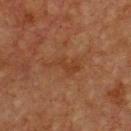Case summary:
* workup: no biopsy performed (imaged during a skin exam)
* lesion size: ≈4.5 mm
* automated metrics: a lesion color around L≈31 a*≈19 b*≈28 in CIELAB and a normalized lesion–skin contrast near 5; border irregularity of about 8 on a 0–10 scale and internal color variation of about 1.5 on a 0–10 scale
* site: the chest
* acquisition: 15 mm crop, total-body photography
* subject: male, about 75 years old
* illumination: cross-polarized